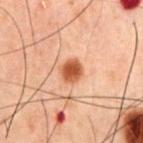Assessment:
No biopsy was performed on this lesion — it was imaged during a full skin examination and was not determined to be concerning.
Clinical summary:
The tile uses cross-polarized illumination. A male subject approximately 60 years of age. From the front of the torso. Measured at roughly 2.5 mm in maximum diameter. A lesion tile, about 15 mm wide, cut from a 3D total-body photograph.